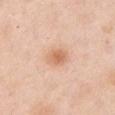Captured during whole-body skin photography for melanoma surveillance; the lesion was not biopsied. A close-up tile cropped from a whole-body skin photograph, about 15 mm across. The subject is a female in their 50s. About 3 mm across. The lesion is located on the chest. The tile uses white-light illumination.